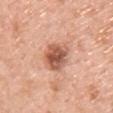Captured under white-light illumination. Located on the chest. Measured at roughly 4 mm in maximum diameter. A male subject aged 58–62. The total-body-photography lesion software estimated border irregularity of about 2 on a 0–10 scale, a color-variation rating of about 6/10, and a peripheral color-asymmetry measure near 1.5. Cropped from a whole-body photographic skin survey; the tile spans about 15 mm.